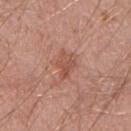{"biopsy_status": "not biopsied; imaged during a skin examination", "image": {"source": "total-body photography crop", "field_of_view_mm": 15}, "site": "left upper arm", "patient": {"sex": "male", "age_approx": 50}, "lesion_size": {"long_diameter_mm_approx": 3.0}}